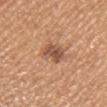Impression:
Recorded during total-body skin imaging; not selected for excision or biopsy.